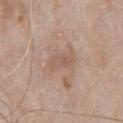follow-up: total-body-photography surveillance lesion; no biopsy | acquisition: 15 mm crop, total-body photography | patient: male, approximately 65 years of age | TBP lesion metrics: an area of roughly 4 mm² and a shape-asymmetry score of about 0.3 (0 = symmetric); a lesion color around L≈56 a*≈18 b*≈27 in CIELAB and roughly 7 lightness units darker than nearby skin | body site: the front of the torso | lesion size: ≈3 mm.